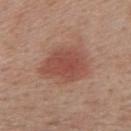Part of a total-body skin-imaging series; this lesion was reviewed on a skin check and was not flagged for biopsy. The lesion is on the upper back. The recorded lesion diameter is about 5.5 mm. Automated tile analysis of the lesion measured a lesion area of about 17 mm² and an outline eccentricity of about 0.7 (0 = round, 1 = elongated). It also reported about 10 CIELAB-L* units darker than the surrounding skin and a normalized border contrast of about 7. And it measured a classifier nevus-likeness of about 100/100 and lesion-presence confidence of about 100/100. A roughly 15 mm field-of-view crop from a total-body skin photograph. This is a white-light tile. A female subject, aged 38–42.